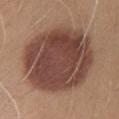Case summary:
• follow-up · imaged on a skin check; not biopsied